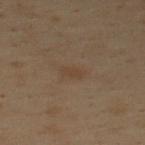The recorded lesion diameter is about 2.5 mm.
The lesion-visualizer software estimated a lesion color around L≈32 a*≈11 b*≈23 in CIELAB and roughly 4 lightness units darker than nearby skin. The software also gave a border-irregularity rating of about 2.5/10, internal color variation of about 1 on a 0–10 scale, and radial color variation of about 0.5.
Cropped from a total-body skin-imaging series; the visible field is about 15 mm.
From the upper back.
A male subject approximately 50 years of age.